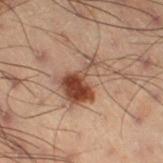Captured during whole-body skin photography for melanoma surveillance; the lesion was not biopsied.
From the right thigh.
Cropped from a total-body skin-imaging series; the visible field is about 15 mm.
A male patient approximately 55 years of age.